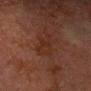Clinical impression: Imaged during a routine full-body skin examination; the lesion was not biopsied and no histopathology is available. Context: Imaged with cross-polarized lighting. The lesion is on the chest. A male patient roughly 75 years of age. A 15 mm crop from a total-body photograph taken for skin-cancer surveillance. An algorithmic analysis of the crop reported a border-irregularity rating of about 4.5/10, internal color variation of about 2.5 on a 0–10 scale, and peripheral color asymmetry of about 1.